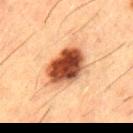| feature | finding |
|---|---|
| workup | total-body-photography surveillance lesion; no biopsy |
| illumination | cross-polarized illumination |
| automated lesion analysis | a footprint of about 16 mm², an eccentricity of roughly 0.75, and a symmetry-axis asymmetry near 0.15; about 20 CIELAB-L* units darker than the surrounding skin and a normalized border contrast of about 14.5 |
| image source | 15 mm crop, total-body photography |
| site | the upper back |
| diameter | ≈5.5 mm |
| patient | male, about 50 years old |